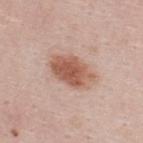Assessment: Captured during whole-body skin photography for melanoma surveillance; the lesion was not biopsied. Acquisition and patient details: The lesion-visualizer software estimated a footprint of about 13 mm², a shape eccentricity near 0.8, and two-axis asymmetry of about 0.15. It also reported a lesion color around L≈57 a*≈22 b*≈29 in CIELAB, about 13 CIELAB-L* units darker than the surrounding skin, and a lesion-to-skin contrast of about 9 (normalized; higher = more distinct). A male subject, about 25 years old. Imaged with white-light lighting. A close-up tile cropped from a whole-body skin photograph, about 15 mm across. Located on the upper back.